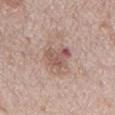Clinical impression: This lesion was catalogued during total-body skin photography and was not selected for biopsy. Acquisition and patient details: A roughly 15 mm field-of-view crop from a total-body skin photograph. On the front of the torso. The total-body-photography lesion software estimated a lesion area of about 7.5 mm², a shape eccentricity near 0.6, and a shape-asymmetry score of about 0.35 (0 = symmetric). It also reported a border-irregularity rating of about 4/10, internal color variation of about 5.5 on a 0–10 scale, and peripheral color asymmetry of about 2. The software also gave a nevus-likeness score of about 0/100 and a lesion-detection confidence of about 100/100. The subject is a male about 70 years old. Approximately 4 mm at its widest. Captured under white-light illumination.